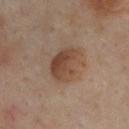Assessment: Imaged during a routine full-body skin examination; the lesion was not biopsied and no histopathology is available. Background: The lesion is on the upper back. The subject is a male aged 43–47. The tile uses cross-polarized illumination. A lesion tile, about 15 mm wide, cut from a 3D total-body photograph.